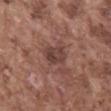Assessment:
Recorded during total-body skin imaging; not selected for excision or biopsy.
Clinical summary:
A 15 mm close-up tile from a total-body photography series done for melanoma screening. The subject is a male aged 73 to 77. From the mid back.